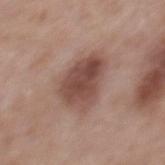Impression:
Part of a total-body skin-imaging series; this lesion was reviewed on a skin check and was not flagged for biopsy.
Context:
The lesion is on the back. The total-body-photography lesion software estimated border irregularity of about 2.5 on a 0–10 scale, a color-variation rating of about 5/10, and radial color variation of about 2. A 15 mm close-up tile from a total-body photography series done for melanoma screening. A male subject, in their mid-50s.